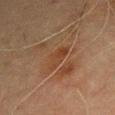This lesion was catalogued during total-body skin photography and was not selected for biopsy. The recorded lesion diameter is about 4 mm. The lesion is on the left upper arm. The tile uses cross-polarized illumination. A 15 mm crop from a total-body photograph taken for skin-cancer surveillance. A male patient, about 70 years old.